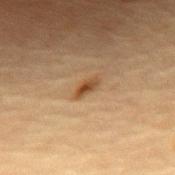<lesion>
  <lesion_size>
    <long_diameter_mm_approx>3.0</long_diameter_mm_approx>
  </lesion_size>
  <patient>
    <sex>female</sex>
    <age_approx>80</age_approx>
  </patient>
  <image>
    <source>total-body photography crop</source>
    <field_of_view_mm>15</field_of_view_mm>
  </image>
  <lighting>cross-polarized</lighting>
  <site>chest</site>
</lesion>